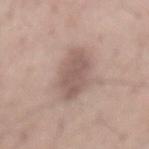Assessment:
No biopsy was performed on this lesion — it was imaged during a full skin examination and was not determined to be concerning.
Acquisition and patient details:
This image is a 15 mm lesion crop taken from a total-body photograph. About 5.5 mm across. A male subject approximately 55 years of age. Located on the mid back. This is a white-light tile.